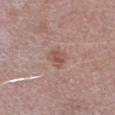No biopsy was performed on this lesion — it was imaged during a full skin examination and was not determined to be concerning.
A 15 mm close-up tile from a total-body photography series done for melanoma screening.
The recorded lesion diameter is about 2.5 mm.
Imaged with white-light lighting.
From the leg.
A male patient, aged 48 to 52.